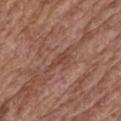Clinical impression: Captured during whole-body skin photography for melanoma surveillance; the lesion was not biopsied. Clinical summary: A male patient about 75 years old. Located on the mid back. A 15 mm close-up extracted from a 3D total-body photography capture.